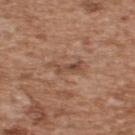The lesion was photographed on a routine skin check and not biopsied; there is no pathology result.
The subject is a male roughly 65 years of age.
The tile uses white-light illumination.
A region of skin cropped from a whole-body photographic capture, roughly 15 mm wide.
Automated tile analysis of the lesion measured an area of roughly 4.5 mm², an eccentricity of roughly 0.9, and a shape-asymmetry score of about 0.45 (0 = symmetric). The software also gave a border-irregularity rating of about 6/10 and internal color variation of about 1.5 on a 0–10 scale. The software also gave a nevus-likeness score of about 0/100 and lesion-presence confidence of about 100/100.
The lesion is located on the back.